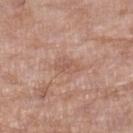workup = total-body-photography surveillance lesion; no biopsy | automated lesion analysis = a mean CIELAB color near L≈56 a*≈21 b*≈27, a lesion–skin lightness drop of about 7, and a normalized border contrast of about 5; a border-irregularity rating of about 2.5/10; an automated nevus-likeness rating near 0 out of 100 and lesion-presence confidence of about 100/100 | location = the left lower leg | patient = female, aged approximately 75 | lesion diameter = about 3 mm | image = ~15 mm tile from a whole-body skin photo | lighting = white-light illumination.